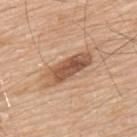Recorded during total-body skin imaging; not selected for excision or biopsy. A lesion tile, about 15 mm wide, cut from a 3D total-body photograph. The lesion is located on the upper back. This is a white-light tile. A male subject approximately 80 years of age. Longest diameter approximately 6.5 mm.